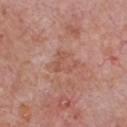Imaged during a routine full-body skin examination; the lesion was not biopsied and no histopathology is available. The recorded lesion diameter is about 3 mm. A lesion tile, about 15 mm wide, cut from a 3D total-body photograph. The patient is a male aged 58–62. From the chest.